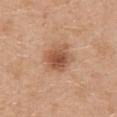automated metrics: a footprint of about 9.5 mm², an outline eccentricity of about 0.4 (0 = round, 1 = elongated), and a symmetry-axis asymmetry near 0.1; an automated nevus-likeness rating near 90 out of 100 and a lesion-detection confidence of about 100/100 | body site: the abdomen | lighting: white-light | patient: female, in their 40s | image: total-body-photography crop, ~15 mm field of view.